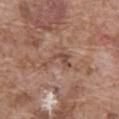Assessment:
Captured during whole-body skin photography for melanoma surveillance; the lesion was not biopsied.
Image and clinical context:
The subject is a male roughly 75 years of age. The lesion-visualizer software estimated an area of roughly 5 mm², an eccentricity of roughly 0.85, and a shape-asymmetry score of about 0.7 (0 = symmetric). The analysis additionally found a mean CIELAB color near L≈49 a*≈20 b*≈26, a lesion–skin lightness drop of about 8, and a lesion-to-skin contrast of about 6 (normalized; higher = more distinct). The analysis additionally found border irregularity of about 9 on a 0–10 scale and a peripheral color-asymmetry measure near 0.5. Approximately 4 mm at its widest. From the abdomen. Imaged with white-light lighting. A 15 mm close-up extracted from a 3D total-body photography capture.